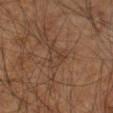<record>
<biopsy_status>not biopsied; imaged during a skin examination</biopsy_status>
<lesion_size>
  <long_diameter_mm_approx>3.5</long_diameter_mm_approx>
</lesion_size>
<site>left arm</site>
<patient>
  <sex>male</sex>
  <age_approx>65</age_approx>
</patient>
<image>
  <source>total-body photography crop</source>
  <field_of_view_mm>15</field_of_view_mm>
</image>
<lighting>cross-polarized</lighting>
</record>